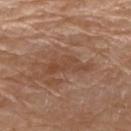This lesion was catalogued during total-body skin photography and was not selected for biopsy. A roughly 15 mm field-of-view crop from a total-body skin photograph. From the chest. The subject is a male aged approximately 80.